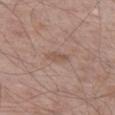<tbp_lesion>
<biopsy_status>not biopsied; imaged during a skin examination</biopsy_status>
<automated_metrics>
  <eccentricity>0.9</eccentricity>
  <shape_asymmetry>0.4</shape_asymmetry>
  <cielab_L>52</cielab_L>
  <cielab_a>18</cielab_a>
  <cielab_b>26</cielab_b>
  <vs_skin_darker_L>7.0</vs_skin_darker_L>
  <vs_skin_contrast_norm>5.5</vs_skin_contrast_norm>
  <lesion_detection_confidence_0_100>100</lesion_detection_confidence_0_100>
</automated_metrics>
<patient>
  <sex>male</sex>
  <age_approx>50</age_approx>
</patient>
<image>
  <source>total-body photography crop</source>
  <field_of_view_mm>15</field_of_view_mm>
</image>
<site>left thigh</site>
<lesion_size>
  <long_diameter_mm_approx>2.5</long_diameter_mm_approx>
</lesion_size>
<lighting>white-light</lighting>
</tbp_lesion>